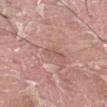Impression: The lesion was photographed on a routine skin check and not biopsied; there is no pathology result. Context: About 2.5 mm across. Captured under white-light illumination. A lesion tile, about 15 mm wide, cut from a 3D total-body photograph. The subject is a male aged 38 to 42. From the left thigh. Automated tile analysis of the lesion measured an area of roughly 3 mm², an eccentricity of roughly 0.85, and a symmetry-axis asymmetry near 0.3. The software also gave an average lesion color of about L≈56 a*≈21 b*≈24 (CIELAB) and a normalized border contrast of about 5.